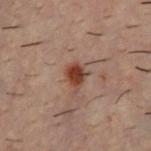{"image": {"source": "total-body photography crop", "field_of_view_mm": 15}, "site": "chest", "automated_metrics": {"vs_skin_contrast_norm": 10.5, "border_irregularity_0_10": 2.0, "color_variation_0_10": 3.0, "peripheral_color_asymmetry": 1.0, "nevus_likeness_0_100": 95, "lesion_detection_confidence_0_100": 100}, "patient": {"sex": "male", "age_approx": 30}}